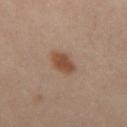Case summary:
* notes: catalogued during a skin exam; not biopsied
* location: the mid back
* patient: male, approximately 40 years of age
* automated metrics: an area of roughly 6.5 mm², an eccentricity of roughly 0.65, and a symmetry-axis asymmetry near 0.2; a classifier nevus-likeness of about 100/100 and a detector confidence of about 100 out of 100 that the crop contains a lesion
* acquisition: 15 mm crop, total-body photography